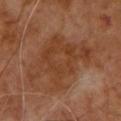biopsy_status: not biopsied; imaged during a skin examination
lighting: cross-polarized
patient:
  sex: male
  age_approx: 65
lesion_size:
  long_diameter_mm_approx: 8.5
image:
  source: total-body photography crop
  field_of_view_mm: 15